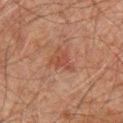This lesion was catalogued during total-body skin photography and was not selected for biopsy. The lesion is on the chest. The tile uses cross-polarized illumination. A male patient, approximately 60 years of age. The total-body-photography lesion software estimated a lesion color around L≈36 a*≈21 b*≈24 in CIELAB and a lesion–skin lightness drop of about 6. The software also gave a border-irregularity index near 5.5/10, a color-variation rating of about 1.5/10, and peripheral color asymmetry of about 0.5. About 3 mm across. This image is a 15 mm lesion crop taken from a total-body photograph.